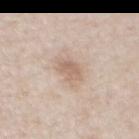No biopsy was performed on this lesion — it was imaged during a full skin examination and was not determined to be concerning. Located on the chest. A 15 mm close-up extracted from a 3D total-body photography capture. A male patient about 60 years old.